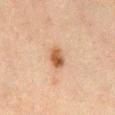  lighting: cross-polarized
  image:
    source: total-body photography crop
    field_of_view_mm: 15
  lesion_size:
    long_diameter_mm_approx: 3.0
  site: abdomen
  patient:
    sex: male
    age_approx: 65